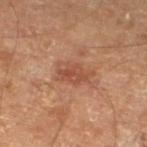Notes:
- biopsy status · catalogued during a skin exam; not biopsied
- image · ~15 mm crop, total-body skin-cancer survey
- automated metrics · a footprint of about 7.5 mm², a shape eccentricity near 0.85, and a symmetry-axis asymmetry near 0.3; a border-irregularity index near 3.5/10, internal color variation of about 3 on a 0–10 scale, and radial color variation of about 1; a classifier nevus-likeness of about 10/100 and lesion-presence confidence of about 100/100
- size · about 4 mm
- lighting · cross-polarized illumination
- location · the leg
- subject · aged approximately 55